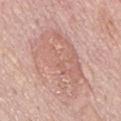<lesion>
<biopsy_status>not biopsied; imaged during a skin examination</biopsy_status>
<image>
  <source>total-body photography crop</source>
  <field_of_view_mm>15</field_of_view_mm>
</image>
<site>mid back</site>
<automated_metrics>
  <area_mm2_approx>34.0</area_mm2_approx>
  <eccentricity>0.9</eccentricity>
  <shape_asymmetry>0.3</shape_asymmetry>
</automated_metrics>
<patient>
  <sex>male</sex>
  <age_approx>75</age_approx>
</patient>
<lesion_size>
  <long_diameter_mm_approx>9.5</long_diameter_mm_approx>
</lesion_size>
</lesion>